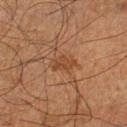<case>
<biopsy_status>not biopsied; imaged during a skin examination</biopsy_status>
<site>left lower leg</site>
<image>
  <source>total-body photography crop</source>
  <field_of_view_mm>15</field_of_view_mm>
</image>
<automated_metrics>
  <area_mm2_approx>3.5</area_mm2_approx>
  <eccentricity>0.85</eccentricity>
  <shape_asymmetry>0.45</shape_asymmetry>
  <cielab_L>36</cielab_L>
  <cielab_a>19</cielab_a>
  <cielab_b>29</cielab_b>
  <vs_skin_darker_L>6.0</vs_skin_darker_L>
  <vs_skin_contrast_norm>6.0</vs_skin_contrast_norm>
  <border_irregularity_0_10>4.5</border_irregularity_0_10>
  <peripheral_color_asymmetry>1.0</peripheral_color_asymmetry>
</automated_metrics>
<patient>
  <sex>male</sex>
  <age_approx>65</age_approx>
</patient>
<lesion_size>
  <long_diameter_mm_approx>3.0</long_diameter_mm_approx>
</lesion_size>
<lighting>cross-polarized</lighting>
</case>